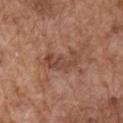notes=no biopsy performed (imaged during a skin exam)
patient=male, aged 73 to 77
diameter=~4 mm (longest diameter)
lighting=white-light illumination
image-analysis metrics=an average lesion color of about L≈45 a*≈22 b*≈28 (CIELAB), roughly 8 lightness units darker than nearby skin, and a normalized border contrast of about 6.5; a border-irregularity rating of about 8/10 and peripheral color asymmetry of about 0.5; a nevus-likeness score of about 0/100 and lesion-presence confidence of about 100/100
image source=~15 mm crop, total-body skin-cancer survey
body site=the right upper arm